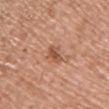Clinical impression: This lesion was catalogued during total-body skin photography and was not selected for biopsy. Acquisition and patient details: A male patient, in their 80s. The total-body-photography lesion software estimated a mean CIELAB color near L≈53 a*≈24 b*≈32, a lesion–skin lightness drop of about 10, and a lesion-to-skin contrast of about 7 (normalized; higher = more distinct). And it measured border irregularity of about 3 on a 0–10 scale, a color-variation rating of about 2.5/10, and a peripheral color-asymmetry measure near 1. The software also gave lesion-presence confidence of about 100/100. Cropped from a total-body skin-imaging series; the visible field is about 15 mm. Approximately 3 mm at its widest. Located on the front of the torso. The tile uses white-light illumination.